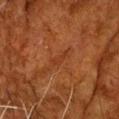Acquisition and patient details: Located on the head or neck. Captured under cross-polarized illumination. Automated tile analysis of the lesion measured a border-irregularity index near 5/10 and peripheral color asymmetry of about 0. A male patient aged 78–82. A region of skin cropped from a whole-body photographic capture, roughly 15 mm wide. The lesion's longest dimension is about 2.5 mm.